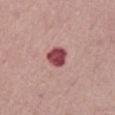{
  "biopsy_status": "not biopsied; imaged during a skin examination",
  "image": {
    "source": "total-body photography crop",
    "field_of_view_mm": 15
  },
  "patient": {
    "sex": "male",
    "age_approx": 75
  },
  "site": "abdomen",
  "lesion_size": {
    "long_diameter_mm_approx": 2.5
  }
}